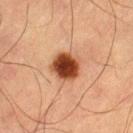The lesion was photographed on a routine skin check and not biopsied; there is no pathology result.
A male subject approximately 65 years of age.
The lesion is on the right thigh.
A region of skin cropped from a whole-body photographic capture, roughly 15 mm wide.